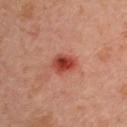workup: no biopsy performed (imaged during a skin exam) | location: the right upper arm | patient: male, approximately 45 years of age | acquisition: total-body-photography crop, ~15 mm field of view | illumination: cross-polarized illumination | size: ~3 mm (longest diameter).